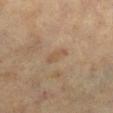No biopsy was performed on this lesion — it was imaged during a full skin examination and was not determined to be concerning.
A female patient aged around 70.
A lesion tile, about 15 mm wide, cut from a 3D total-body photograph.
Located on the right lower leg.